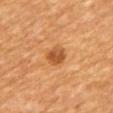Impression: Imaged during a routine full-body skin examination; the lesion was not biopsied and no histopathology is available. Background: The tile uses cross-polarized illumination. Located on the mid back. A close-up tile cropped from a whole-body skin photograph, about 15 mm across. A female subject, in their mid-60s. Longest diameter approximately 2.5 mm.